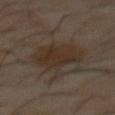Findings:
– follow-up: total-body-photography surveillance lesion; no biopsy
– illumination: cross-polarized
– site: the front of the torso
– subject: male, approximately 60 years of age
– automated lesion analysis: a border-irregularity rating of about 4/10, a within-lesion color-variation index near 3/10, and peripheral color asymmetry of about 1; a nevus-likeness score of about 35/100
– imaging modality: 15 mm crop, total-body photography